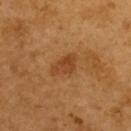Q: Is there a histopathology result?
A: no biopsy performed (imaged during a skin exam)
Q: How was this image acquired?
A: total-body-photography crop, ~15 mm field of view
Q: Where on the body is the lesion?
A: the upper back
Q: Patient demographics?
A: female, approximately 55 years of age
Q: What did automated image analysis measure?
A: an outline eccentricity of about 0.8 (0 = round, 1 = elongated) and a symmetry-axis asymmetry near 0.35; an average lesion color of about L≈44 a*≈25 b*≈40 (CIELAB) and a normalized lesion–skin contrast near 6.5; a color-variation rating of about 3/10 and a peripheral color-asymmetry measure near 1
Q: How was the tile lit?
A: cross-polarized